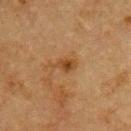The lesion was photographed on a routine skin check and not biopsied; there is no pathology result.
The patient is a male in their mid-70s.
From the front of the torso.
This image is a 15 mm lesion crop taken from a total-body photograph.